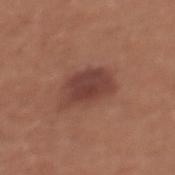Imaged during a routine full-body skin examination; the lesion was not biopsied and no histopathology is available.
A female subject aged 33–37.
Approximately 5 mm at its widest.
From the chest.
A 15 mm crop from a total-body photograph taken for skin-cancer surveillance.